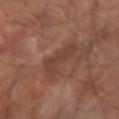This lesion was catalogued during total-body skin photography and was not selected for biopsy.
This image is a 15 mm lesion crop taken from a total-body photograph.
Located on the right forearm.
Longest diameter approximately 4.5 mm.
The total-body-photography lesion software estimated an area of roughly 8.5 mm².
The subject is a male aged 68 to 72.